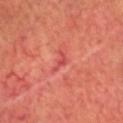Case summary:
• workup — imaged on a skin check; not biopsied
• diameter — about 3 mm
• location — the head or neck
• image — ~15 mm crop, total-body skin-cancer survey
• patient — male, aged 63–67
• lighting — cross-polarized illumination